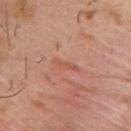follow-up — no biopsy performed (imaged during a skin exam)
image source — 15 mm crop, total-body photography
subject — male, approximately 40 years of age
lesion diameter — about 3 mm
anatomic site — the upper back
tile lighting — cross-polarized